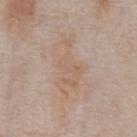No biopsy was performed on this lesion — it was imaged during a full skin examination and was not determined to be concerning. A male subject, roughly 75 years of age. From the chest. Longest diameter approximately 7 mm. A region of skin cropped from a whole-body photographic capture, roughly 15 mm wide. Automated image analysis of the tile measured a border-irregularity rating of about 8/10, internal color variation of about 2.5 on a 0–10 scale, and radial color variation of about 0.5. And it measured a lesion-detection confidence of about 100/100.